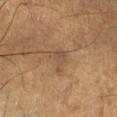Recorded during total-body skin imaging; not selected for excision or biopsy. A male subject aged 53–57. Imaged with cross-polarized lighting. The recorded lesion diameter is about 3 mm. Automated tile analysis of the lesion measured an eccentricity of roughly 0.65 and a shape-asymmetry score of about 0.45 (0 = symmetric). And it measured a border-irregularity rating of about 4.5/10, internal color variation of about 2 on a 0–10 scale, and radial color variation of about 0.5. On the right lower leg. Cropped from a total-body skin-imaging series; the visible field is about 15 mm.